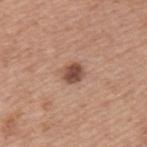Q: Was a biopsy performed?
A: imaged on a skin check; not biopsied
Q: Who is the patient?
A: male, in their mid- to late 70s
Q: Automated lesion metrics?
A: an average lesion color of about L≈50 a*≈21 b*≈27 (CIELAB), roughly 13 lightness units darker than nearby skin, and a normalized border contrast of about 9.5
Q: How was the tile lit?
A: white-light
Q: How large is the lesion?
A: ~2.5 mm (longest diameter)
Q: What is the imaging modality?
A: total-body-photography crop, ~15 mm field of view
Q: What is the anatomic site?
A: the left upper arm